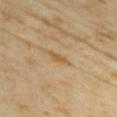Case summary:
- biopsy status — total-body-photography surveillance lesion; no biopsy
- image source — ~15 mm tile from a whole-body skin photo
- location — the upper back
- subject — female, aged approximately 35